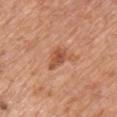• workup: total-body-photography surveillance lesion; no biopsy
• body site: the front of the torso
• diameter: ~3.5 mm (longest diameter)
• tile lighting: white-light
• image source: ~15 mm tile from a whole-body skin photo
• TBP lesion metrics: a border-irregularity rating of about 3.5/10, a within-lesion color-variation index near 3/10, and a peripheral color-asymmetry measure near 1
• subject: male, in their 60s